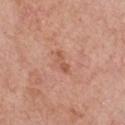Recorded during total-body skin imaging; not selected for excision or biopsy. A male patient, about 80 years old. This image is a 15 mm lesion crop taken from a total-body photograph. On the front of the torso. The recorded lesion diameter is about 3 mm. Automated tile analysis of the lesion measured a nevus-likeness score of about 0/100 and a lesion-detection confidence of about 100/100.